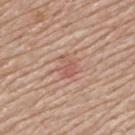Assessment:
Imaged during a routine full-body skin examination; the lesion was not biopsied and no histopathology is available.
Background:
Captured under white-light illumination. Cropped from a whole-body photographic skin survey; the tile spans about 15 mm. The lesion-visualizer software estimated a mean CIELAB color near L≈57 a*≈24 b*≈27, about 7 CIELAB-L* units darker than the surrounding skin, and a normalized lesion–skin contrast near 5. From the upper back. About 2.5 mm across. A male subject aged 78 to 82.